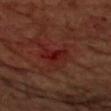Imaged during a routine full-body skin examination; the lesion was not biopsied and no histopathology is available. A region of skin cropped from a whole-body photographic capture, roughly 15 mm wide. The patient is a male about 65 years old. Located on the left upper arm.